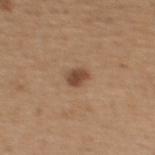Captured during whole-body skin photography for melanoma surveillance; the lesion was not biopsied. The lesion's longest dimension is about 2.5 mm. This is a white-light tile. A 15 mm close-up extracted from a 3D total-body photography capture. A female subject, aged approximately 50. The lesion is located on the upper back.